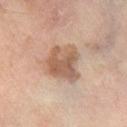Clinical impression:
Imaged during a routine full-body skin examination; the lesion was not biopsied and no histopathology is available.
Image and clinical context:
A region of skin cropped from a whole-body photographic capture, roughly 15 mm wide. The tile uses cross-polarized illumination. A male patient, about 60 years old. About 4.5 mm across. From the right lower leg.